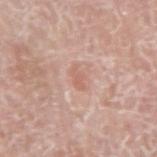Q: Is there a histopathology result?
A: total-body-photography surveillance lesion; no biopsy
Q: How was the tile lit?
A: white-light illumination
Q: Patient demographics?
A: male, in their mid- to late 70s
Q: Where on the body is the lesion?
A: the right upper arm
Q: How was this image acquired?
A: total-body-photography crop, ~15 mm field of view
Q: Lesion size?
A: about 2.5 mm